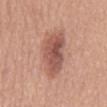Q: Was this lesion biopsied?
A: total-body-photography surveillance lesion; no biopsy
Q: Who is the patient?
A: male, aged 63–67
Q: What is the lesion's diameter?
A: ~7 mm (longest diameter)
Q: What kind of image is this?
A: 15 mm crop, total-body photography
Q: Where on the body is the lesion?
A: the abdomen
Q: Automated lesion metrics?
A: a mean CIELAB color near L≈54 a*≈23 b*≈27, about 12 CIELAB-L* units darker than the surrounding skin, and a lesion-to-skin contrast of about 8 (normalized; higher = more distinct); a classifier nevus-likeness of about 85/100 and a detector confidence of about 100 out of 100 that the crop contains a lesion
Q: How was the tile lit?
A: white-light